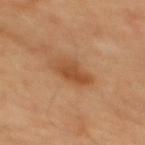workup: catalogued during a skin exam; not biopsied | anatomic site: the back | imaging modality: ~15 mm crop, total-body skin-cancer survey | automated metrics: an outline eccentricity of about 0.75 (0 = round, 1 = elongated) and a shape-asymmetry score of about 0.25 (0 = symmetric) | subject: male, aged approximately 65 | tile lighting: cross-polarized.